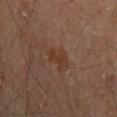Impression: The lesion was tiled from a total-body skin photograph and was not biopsied. Clinical summary: A lesion tile, about 15 mm wide, cut from a 3D total-body photograph. A male patient in their mid- to late 60s. From the left upper arm.